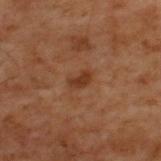follow-up = imaged on a skin check; not biopsied.